The lesion's longest dimension is about 2.5 mm, from the right thigh, a female subject aged approximately 40, a 15 mm close-up extracted from a 3D total-body photography capture, this is a cross-polarized tile — 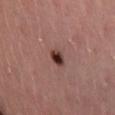Conclusion: Histopathology of the biopsied lesion showed a benign skin lesion: junctional melanocytic nevus.